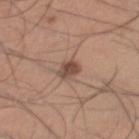Clinical impression: The lesion was photographed on a routine skin check and not biopsied; there is no pathology result. Background: A region of skin cropped from a whole-body photographic capture, roughly 15 mm wide. Automated tile analysis of the lesion measured a color-variation rating of about 2.5/10 and peripheral color asymmetry of about 1. Located on the right lower leg. A male subject aged around 35. Measured at roughly 2.5 mm in maximum diameter.